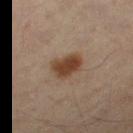No biopsy was performed on this lesion — it was imaged during a full skin examination and was not determined to be concerning. Located on the right thigh. A roughly 15 mm field-of-view crop from a total-body skin photograph. An algorithmic analysis of the crop reported a lesion area of about 7.5 mm², a shape eccentricity near 0.75, and two-axis asymmetry of about 0.2. The analysis additionally found an average lesion color of about L≈41 a*≈18 b*≈29 (CIELAB), about 12 CIELAB-L* units darker than the surrounding skin, and a lesion-to-skin contrast of about 10.5 (normalized; higher = more distinct). It also reported internal color variation of about 3 on a 0–10 scale and radial color variation of about 1. The patient is a male in their mid- to late 50s. Captured under cross-polarized illumination. About 3.5 mm across.